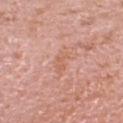No biopsy was performed on this lesion — it was imaged during a full skin examination and was not determined to be concerning.